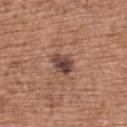follow-up: catalogued during a skin exam; not biopsied
acquisition: ~15 mm tile from a whole-body skin photo
site: the back
patient: female, approximately 60 years of age